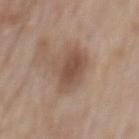notes=no biopsy performed (imaged during a skin exam)